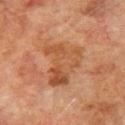<record>
<biopsy_status>not biopsied; imaged during a skin examination</biopsy_status>
<lighting>cross-polarized</lighting>
<automated_metrics>
  <area_mm2_approx>19.0</area_mm2_approx>
  <eccentricity>0.65</eccentricity>
  <shape_asymmetry>0.45</shape_asymmetry>
  <border_irregularity_0_10>5.0</border_irregularity_0_10>
  <color_variation_0_10>5.5</color_variation_0_10>
  <peripheral_color_asymmetry>1.5</peripheral_color_asymmetry>
  <nevus_likeness_0_100>0</nevus_likeness_0_100>
</automated_metrics>
<site>left upper arm</site>
<image>
  <source>total-body photography crop</source>
  <field_of_view_mm>15</field_of_view_mm>
</image>
<patient>
  <sex>male</sex>
  <age_approx>75</age_approx>
</patient>
</record>